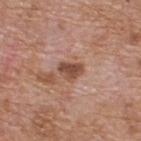Assessment:
The lesion was tiled from a total-body skin photograph and was not biopsied.
Acquisition and patient details:
This is a white-light tile. An algorithmic analysis of the crop reported an area of roughly 5 mm², an outline eccentricity of about 0.75 (0 = round, 1 = elongated), and two-axis asymmetry of about 0.35. And it measured border irregularity of about 3 on a 0–10 scale, internal color variation of about 3 on a 0–10 scale, and peripheral color asymmetry of about 1. The analysis additionally found a classifier nevus-likeness of about 5/100 and lesion-presence confidence of about 100/100. About 3 mm across. The lesion is on the upper back. The subject is a male about 60 years old. A 15 mm crop from a total-body photograph taken for skin-cancer surveillance.